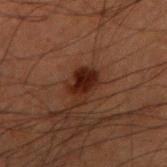Clinical impression: Imaged during a routine full-body skin examination; the lesion was not biopsied and no histopathology is available. Image and clinical context: The tile uses cross-polarized illumination. Cropped from a total-body skin-imaging series; the visible field is about 15 mm. A male patient, aged 58 to 62. The lesion is on the right upper arm. Automated image analysis of the tile measured an area of roughly 6.5 mm². The software also gave an average lesion color of about L≈17 a*≈18 b*≈20 (CIELAB), roughly 9 lightness units darker than nearby skin, and a normalized lesion–skin contrast near 11. It also reported border irregularity of about 3.5 on a 0–10 scale, internal color variation of about 4 on a 0–10 scale, and peripheral color asymmetry of about 1.5. The software also gave a classifier nevus-likeness of about 90/100 and a lesion-detection confidence of about 100/100. About 3 mm across.